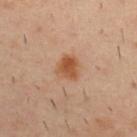Recorded during total-body skin imaging; not selected for excision or biopsy. Approximately 3 mm at its widest. The lesion is on the upper back. Automated tile analysis of the lesion measured a mean CIELAB color near L≈52 a*≈23 b*≈35 and a lesion-to-skin contrast of about 8.5 (normalized; higher = more distinct). The software also gave an automated nevus-likeness rating near 100 out of 100 and lesion-presence confidence of about 100/100. A male patient aged around 40. This is a cross-polarized tile. A 15 mm crop from a total-body photograph taken for skin-cancer surveillance.